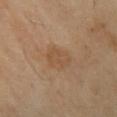Findings:
• follow-up: catalogued during a skin exam; not biopsied
• location: the right lower leg
• acquisition: 15 mm crop, total-body photography
• patient: female, aged 68–72